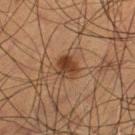Clinical impression: Imaged during a routine full-body skin examination; the lesion was not biopsied and no histopathology is available. Image and clinical context: The lesion's longest dimension is about 3 mm. The lesion is on the left thigh. Captured under cross-polarized illumination. A lesion tile, about 15 mm wide, cut from a 3D total-body photograph. The subject is a male aged around 50. An algorithmic analysis of the crop reported a mean CIELAB color near L≈31 a*≈17 b*≈26 and about 9 CIELAB-L* units darker than the surrounding skin. The analysis additionally found a border-irregularity index near 2/10 and a peripheral color-asymmetry measure near 1.5. And it measured a nevus-likeness score of about 85/100 and a detector confidence of about 100 out of 100 that the crop contains a lesion.